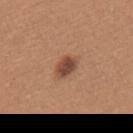Q: Was this lesion biopsied?
A: imaged on a skin check; not biopsied
Q: How was the tile lit?
A: white-light illumination
Q: Who is the patient?
A: female, roughly 40 years of age
Q: What did automated image analysis measure?
A: a footprint of about 5.5 mm² and a shape eccentricity near 0.75; a normalized lesion–skin contrast near 9; a nevus-likeness score of about 90/100 and a detector confidence of about 100 out of 100 that the crop contains a lesion
Q: Lesion location?
A: the upper back
Q: How large is the lesion?
A: about 3 mm
Q: What is the imaging modality?
A: ~15 mm crop, total-body skin-cancer survey